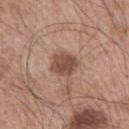The total-body-photography lesion software estimated a lesion area of about 7.5 mm², an outline eccentricity of about 0.5 (0 = round, 1 = elongated), and a symmetry-axis asymmetry near 0.15. And it measured roughly 12 lightness units darker than nearby skin and a lesion-to-skin contrast of about 8.5 (normalized; higher = more distinct). The analysis additionally found a within-lesion color-variation index near 3.5/10 and peripheral color asymmetry of about 1. Imaged with white-light lighting. The lesion is located on the left upper arm. The subject is a male in their mid-60s. Cropped from a total-body skin-imaging series; the visible field is about 15 mm.